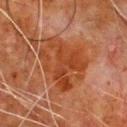Impression: No biopsy was performed on this lesion — it was imaged during a full skin examination and was not determined to be concerning. Acquisition and patient details: Captured under cross-polarized illumination. This image is a 15 mm lesion crop taken from a total-body photograph. A male subject approximately 80 years of age. About 6.5 mm across. Located on the front of the torso.